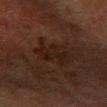{
  "biopsy_status": "not biopsied; imaged during a skin examination",
  "patient": {
    "sex": "male",
    "age_approx": 65
  },
  "image": {
    "source": "total-body photography crop",
    "field_of_view_mm": 15
  },
  "site": "right forearm"
}